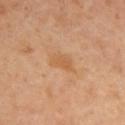Findings:
- follow-up — total-body-photography surveillance lesion; no biopsy
- diameter — ≈3.5 mm
- subject — female, aged around 40
- lighting — cross-polarized
- imaging modality — total-body-photography crop, ~15 mm field of view
- anatomic site — the chest